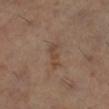Q: Was a biopsy performed?
A: total-body-photography surveillance lesion; no biopsy
Q: How large is the lesion?
A: ~3.5 mm (longest diameter)
Q: What is the imaging modality?
A: total-body-photography crop, ~15 mm field of view
Q: Where on the body is the lesion?
A: the right lower leg
Q: Who is the patient?
A: female, roughly 60 years of age
Q: How was the tile lit?
A: cross-polarized